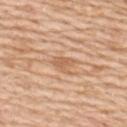follow-up — imaged on a skin check; not biopsied | illumination — white-light illumination | location — the upper back | diameter — ~3 mm (longest diameter) | TBP lesion metrics — a lesion color around L≈61 a*≈21 b*≈36 in CIELAB, roughly 9 lightness units darker than nearby skin, and a lesion-to-skin contrast of about 6 (normalized; higher = more distinct); border irregularity of about 4 on a 0–10 scale and a color-variation rating of about 0.5/10; an automated nevus-likeness rating near 0 out of 100 and lesion-presence confidence of about 80/100 | acquisition — 15 mm crop, total-body photography | subject — male, aged around 60.